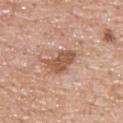biopsy status: imaged on a skin check; not biopsied | acquisition: ~15 mm tile from a whole-body skin photo | patient: male, about 50 years old | diameter: ≈4 mm | body site: the upper back | illumination: white-light | automated metrics: a footprint of about 7.5 mm², a shape eccentricity near 0.75, and a shape-asymmetry score of about 0.3 (0 = symmetric); a mean CIELAB color near L≈54 a*≈22 b*≈31 and roughly 12 lightness units darker than nearby skin; a nevus-likeness score of about 5/100 and a detector confidence of about 100 out of 100 that the crop contains a lesion.